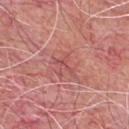notes = total-body-photography surveillance lesion; no biopsy | image = 15 mm crop, total-body photography | site = the front of the torso | patient = male, approximately 60 years of age | image-analysis metrics = a lesion area of about 1.5 mm² and two-axis asymmetry of about 0.55; a mean CIELAB color near L≈51 a*≈31 b*≈24, roughly 7 lightness units darker than nearby skin, and a normalized lesion–skin contrast near 5.